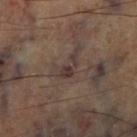biopsy_status: not biopsied; imaged during a skin examination
image:
  source: total-body photography crop
  field_of_view_mm: 15
site: right lower leg
automated_metrics:
  cielab_L: 35
  cielab_a: 14
  cielab_b: 18
  vs_skin_darker_L: 8.0
  vs_skin_contrast_norm: 8.0
  border_irregularity_0_10: 7.0
  color_variation_0_10: 1.5
  peripheral_color_asymmetry: 0.5